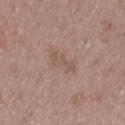Assessment:
Recorded during total-body skin imaging; not selected for excision or biopsy.
Image and clinical context:
A male patient, aged around 50. The lesion's longest dimension is about 4 mm. A 15 mm crop from a total-body photograph taken for skin-cancer surveillance. This is a white-light tile. From the leg.